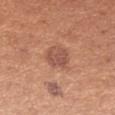This lesion was catalogued during total-body skin photography and was not selected for biopsy.
Longest diameter approximately 3.5 mm.
Located on the right forearm.
A region of skin cropped from a whole-body photographic capture, roughly 15 mm wide.
Captured under white-light illumination.
The patient is a female in their mid- to late 20s.